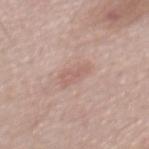Clinical impression:
The lesion was photographed on a routine skin check and not biopsied; there is no pathology result.
Image and clinical context:
A male patient, in their mid- to late 50s. An algorithmic analysis of the crop reported a color-variation rating of about 1.5/10 and peripheral color asymmetry of about 0.5. And it measured a classifier nevus-likeness of about 0/100 and a detector confidence of about 100 out of 100 that the crop contains a lesion. The tile uses white-light illumination. Measured at roughly 3.5 mm in maximum diameter. On the mid back. A 15 mm crop from a total-body photograph taken for skin-cancer surveillance.